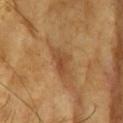Clinical impression:
The lesion was photographed on a routine skin check and not biopsied; there is no pathology result.
Image and clinical context:
The lesion-visualizer software estimated a color-variation rating of about 3/10 and peripheral color asymmetry of about 1. It also reported a nevus-likeness score of about 10/100 and a detector confidence of about 95 out of 100 that the crop contains a lesion. Longest diameter approximately 4 mm. Imaged with cross-polarized lighting. The patient is a female aged approximately 60. From the head or neck. This image is a 15 mm lesion crop taken from a total-body photograph.